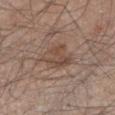Findings:
– notes · no biopsy performed (imaged during a skin exam)
– lesion diameter · about 4.5 mm
– location · the left lower leg
– illumination · white-light
– image-analysis metrics · an outline eccentricity of about 0.8 (0 = round, 1 = elongated) and a symmetry-axis asymmetry near 0.35
– subject · male, aged approximately 45
– imaging modality · ~15 mm crop, total-body skin-cancer survey